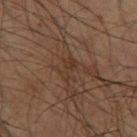Notes:
- notes · catalogued during a skin exam; not biopsied
- automated metrics · a footprint of about 4.5 mm² and two-axis asymmetry of about 0.35
- illumination · cross-polarized
- lesion size · ≈3 mm
- acquisition · total-body-photography crop, ~15 mm field of view
- patient · male, roughly 60 years of age
- site · the right thigh